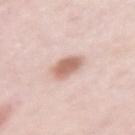Findings:
- biopsy status: total-body-photography surveillance lesion; no biopsy
- body site: the left upper arm
- lesion size: ~3 mm (longest diameter)
- subject: female, aged approximately 65
- imaging modality: 15 mm crop, total-body photography
- lighting: white-light
- automated metrics: a border-irregularity rating of about 2/10, internal color variation of about 2.5 on a 0–10 scale, and peripheral color asymmetry of about 1; a nevus-likeness score of about 90/100 and a detector confidence of about 100 out of 100 that the crop contains a lesion